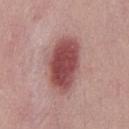lesion diameter = about 7 mm | image source = ~15 mm crop, total-body skin-cancer survey | patient = male, aged approximately 30.